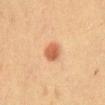biopsy status = imaged on a skin check; not biopsied | location = the abdomen | illumination = cross-polarized illumination | diameter = about 2.5 mm | subject = female, about 40 years old | imaging modality = ~15 mm tile from a whole-body skin photo.